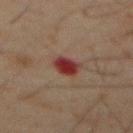Impression: Captured during whole-body skin photography for melanoma surveillance; the lesion was not biopsied. Clinical summary: On the abdomen. The lesion-visualizer software estimated a border-irregularity index near 1.5/10, internal color variation of about 4 on a 0–10 scale, and peripheral color asymmetry of about 1. The subject is a male aged around 60. Measured at roughly 2.5 mm in maximum diameter. A close-up tile cropped from a whole-body skin photograph, about 15 mm across.